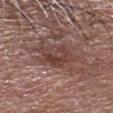biopsy status = no biopsy performed (imaged during a skin exam); image = ~15 mm crop, total-body skin-cancer survey; location = the head or neck; subject = male, in their 80s; illumination = white-light.